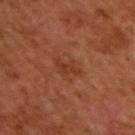* follow-up: catalogued during a skin exam; not biopsied
* size: about 3 mm
* illumination: cross-polarized illumination
* acquisition: ~15 mm crop, total-body skin-cancer survey
* site: the upper back
* patient: male, aged approximately 50